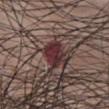{
  "biopsy_status": "not biopsied; imaged during a skin examination",
  "image": {
    "source": "total-body photography crop",
    "field_of_view_mm": 15
  },
  "patient": {
    "sex": "male",
    "age_approx": 50
  },
  "automated_metrics": {
    "area_mm2_approx": 6.5,
    "eccentricity": 0.85,
    "shape_asymmetry": 0.3,
    "nevus_likeness_0_100": 75,
    "lesion_detection_confidence_0_100": 100
  },
  "site": "chest",
  "lighting": "white-light"
}